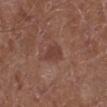| field | value |
|---|---|
| follow-up | total-body-photography surveillance lesion; no biopsy |
| acquisition | ~15 mm tile from a whole-body skin photo |
| subject | male, about 75 years old |
| site | the left lower leg |
| size | ~3 mm (longest diameter) |